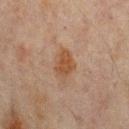Assessment: Captured during whole-body skin photography for melanoma surveillance; the lesion was not biopsied. Context: The lesion-visualizer software estimated a lesion area of about 6.5 mm², a shape eccentricity near 0.75, and a symmetry-axis asymmetry near 0.35. The software also gave border irregularity of about 3.5 on a 0–10 scale and peripheral color asymmetry of about 0.5. The analysis additionally found a nevus-likeness score of about 75/100 and lesion-presence confidence of about 100/100. This image is a 15 mm lesion crop taken from a total-body photograph. Longest diameter approximately 3.5 mm. A male subject about 65 years old. The lesion is on the mid back. Captured under cross-polarized illumination.